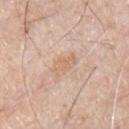Part of a total-body skin-imaging series; this lesion was reviewed on a skin check and was not flagged for biopsy.
Located on the chest.
A male patient, about 70 years old.
The recorded lesion diameter is about 3 mm.
A lesion tile, about 15 mm wide, cut from a 3D total-body photograph.
This is a white-light tile.